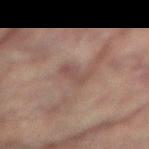Notes:
- biopsy status · catalogued during a skin exam; not biopsied
- patient · female, aged around 80
- anatomic site · the left leg
- image source · ~15 mm tile from a whole-body skin photo
- automated metrics · about 6 CIELAB-L* units darker than the surrounding skin and a normalized lesion–skin contrast near 5.5